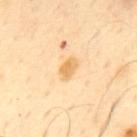Part of a total-body skin-imaging series; this lesion was reviewed on a skin check and was not flagged for biopsy.
A male patient, aged 63 to 67.
This is a cross-polarized tile.
A 15 mm close-up tile from a total-body photography series done for melanoma screening.
The lesion is located on the upper back.
The lesion's longest dimension is about 2.5 mm.